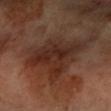Impression:
Part of a total-body skin-imaging series; this lesion was reviewed on a skin check and was not flagged for biopsy.
Clinical summary:
The subject is a female in their 60s. A lesion tile, about 15 mm wide, cut from a 3D total-body photograph. The lesion is located on the left forearm.